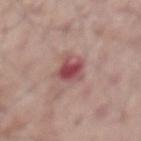body site: the front of the torso
TBP lesion metrics: an average lesion color of about L≈49 a*≈27 b*≈20 (CIELAB), a lesion–skin lightness drop of about 13, and a normalized lesion–skin contrast near 9; border irregularity of about 4 on a 0–10 scale and a peripheral color-asymmetry measure near 1
subject: male, about 65 years old
illumination: white-light
imaging modality: ~15 mm crop, total-body skin-cancer survey
size: ≈3.5 mm